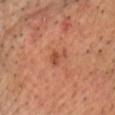Findings:
– tile lighting · cross-polarized
– image source · total-body-photography crop, ~15 mm field of view
– site · the chest
– image-analysis metrics · an average lesion color of about L≈49 a*≈27 b*≈34 (CIELAB), a lesion–skin lightness drop of about 9, and a normalized lesion–skin contrast near 6.5; border irregularity of about 5 on a 0–10 scale and internal color variation of about 1 on a 0–10 scale; lesion-presence confidence of about 100/100
– subject · male, about 70 years old